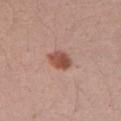Q: Was a biopsy performed?
A: catalogued during a skin exam; not biopsied
Q: Patient demographics?
A: male, aged around 40
Q: How was this image acquired?
A: total-body-photography crop, ~15 mm field of view
Q: Lesion location?
A: the right upper arm